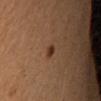Clinical impression:
This lesion was catalogued during total-body skin photography and was not selected for biopsy.
Context:
A male patient aged approximately 45. This is a cross-polarized tile. The lesion is located on the arm. The lesion's longest dimension is about 1.5 mm. The lesion-visualizer software estimated a lesion area of about 2 mm². The analysis additionally found a mean CIELAB color near L≈31 a*≈19 b*≈26. And it measured a classifier nevus-likeness of about 95/100 and lesion-presence confidence of about 100/100. A close-up tile cropped from a whole-body skin photograph, about 15 mm across.